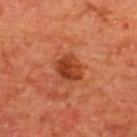The lesion was photographed on a routine skin check and not biopsied; there is no pathology result.
Imaged with cross-polarized lighting.
A roughly 15 mm field-of-view crop from a total-body skin photograph.
Located on the upper back.
The patient is a male about 65 years old.